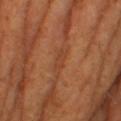Imaged during a routine full-body skin examination; the lesion was not biopsied and no histopathology is available.
Automated image analysis of the tile measured an area of roughly 3 mm². And it measured a nevus-likeness score of about 0/100 and a lesion-detection confidence of about 80/100.
The recorded lesion diameter is about 2.5 mm.
A lesion tile, about 15 mm wide, cut from a 3D total-body photograph.
A female subject in their mid- to late 50s.
On the right lower leg.